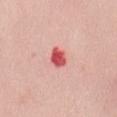notes — catalogued during a skin exam; not biopsied | TBP lesion metrics — a footprint of about 4.5 mm², an eccentricity of roughly 0.65, and a symmetry-axis asymmetry near 0.2; a color-variation rating of about 4/10 and peripheral color asymmetry of about 1.5; a classifier nevus-likeness of about 0/100 and a lesion-detection confidence of about 100/100 | body site — the mid back | imaging modality — ~15 mm crop, total-body skin-cancer survey | subject — female, roughly 40 years of age | diameter — ≈3 mm.